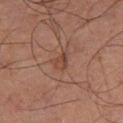notes = catalogued during a skin exam; not biopsied | diameter = about 2.5 mm | TBP lesion metrics = a mean CIELAB color near L≈35 a*≈18 b*≈23, a lesion–skin lightness drop of about 6, and a normalized border contrast of about 5.5; a nevus-likeness score of about 0/100 | tile lighting = cross-polarized illumination | imaging modality = 15 mm crop, total-body photography | anatomic site = the right thigh | subject = male, in their mid-60s.